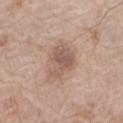A lesion tile, about 15 mm wide, cut from a 3D total-body photograph. A female subject, about 75 years old. Captured under white-light illumination. The total-body-photography lesion software estimated a classifier nevus-likeness of about 15/100 and a lesion-detection confidence of about 100/100. The lesion is on the arm.